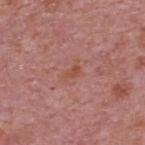No biopsy was performed on this lesion — it was imaged during a full skin examination and was not determined to be concerning. The patient is a male aged 73 to 77. This is a white-light tile. A 15 mm crop from a total-body photograph taken for skin-cancer surveillance. Measured at roughly 3 mm in maximum diameter. The lesion is located on the upper back.